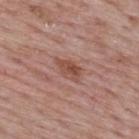Clinical impression: Part of a total-body skin-imaging series; this lesion was reviewed on a skin check and was not flagged for biopsy. Context: Located on the upper back. A male patient about 65 years old. Longest diameter approximately 3 mm. A 15 mm close-up tile from a total-body photography series done for melanoma screening.